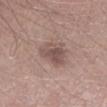patient = male, aged around 55
location = the left lower leg
image = ~15 mm tile from a whole-body skin photo
illumination = white-light illumination
diameter = ~4 mm (longest diameter)